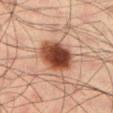Assessment: Captured during whole-body skin photography for melanoma surveillance; the lesion was not biopsied. Context: A male subject, aged around 35. A close-up tile cropped from a whole-body skin photograph, about 15 mm across. The recorded lesion diameter is about 5 mm. This is a cross-polarized tile. On the left lower leg.